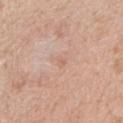Clinical impression: Captured during whole-body skin photography for melanoma surveillance; the lesion was not biopsied. Background: A female subject aged 48 to 52. The lesion is located on the right upper arm. This is a white-light tile. A 15 mm close-up tile from a total-body photography series done for melanoma screening. Longest diameter approximately 1.5 mm.